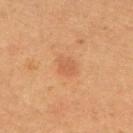Impression:
Part of a total-body skin-imaging series; this lesion was reviewed on a skin check and was not flagged for biopsy.
Image and clinical context:
The lesion is on the upper back. The recorded lesion diameter is about 3 mm. An algorithmic analysis of the crop reported a lesion color around L≈48 a*≈21 b*≈32 in CIELAB and about 6 CIELAB-L* units darker than the surrounding skin. A female subject about 40 years old. This image is a 15 mm lesion crop taken from a total-body photograph. The tile uses cross-polarized illumination.